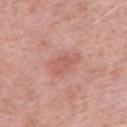Case summary:
* biopsy status: no biopsy performed (imaged during a skin exam)
* anatomic site: the arm
* image-analysis metrics: a border-irregularity rating of about 4/10, a color-variation rating of about 1.5/10, and radial color variation of about 0.5; an automated nevus-likeness rating near 5 out of 100 and a lesion-detection confidence of about 100/100
* image: ~15 mm crop, total-body skin-cancer survey
* diameter: ~3.5 mm (longest diameter)
* tile lighting: white-light illumination
* subject: female, in their mid- to late 40s